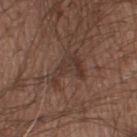Impression: Recorded during total-body skin imaging; not selected for excision or biopsy. Context: About 4 mm across. Captured under white-light illumination. A region of skin cropped from a whole-body photographic capture, roughly 15 mm wide. A male patient aged around 20. Automated image analysis of the tile measured a footprint of about 6.5 mm². And it measured an automated nevus-likeness rating near 0 out of 100. On the right thigh.